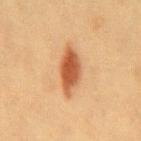This lesion was catalogued during total-body skin photography and was not selected for biopsy.
A 15 mm close-up tile from a total-body photography series done for melanoma screening.
A female patient aged 38 to 42.
Automated tile analysis of the lesion measured an average lesion color of about L≈49 a*≈24 b*≈35 (CIELAB), roughly 14 lightness units darker than nearby skin, and a normalized border contrast of about 9.5. And it measured a classifier nevus-likeness of about 100/100 and lesion-presence confidence of about 100/100.
The lesion's longest dimension is about 5.5 mm.
The tile uses cross-polarized illumination.
The lesion is on the mid back.